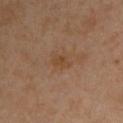Assessment: Imaged during a routine full-body skin examination; the lesion was not biopsied and no histopathology is available. Acquisition and patient details: On the chest. The subject is a male aged 43 to 47. The tile uses cross-polarized illumination. A roughly 15 mm field-of-view crop from a total-body skin photograph. Automated image analysis of the tile measured an outline eccentricity of about 0.8 (0 = round, 1 = elongated) and two-axis asymmetry of about 0.3. The software also gave an average lesion color of about L≈43 a*≈18 b*≈32 (CIELAB), roughly 6 lightness units darker than nearby skin, and a normalized lesion–skin contrast near 6.5. The software also gave a border-irregularity index near 3/10, a within-lesion color-variation index near 1.5/10, and a peripheral color-asymmetry measure near 0.5. And it measured a classifier nevus-likeness of about 0/100 and a detector confidence of about 100 out of 100 that the crop contains a lesion. Longest diameter approximately 2.5 mm.